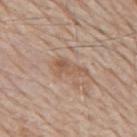A roughly 15 mm field-of-view crop from a total-body skin photograph. The lesion is on the mid back. A male patient, aged approximately 80. Approximately 4.5 mm at its widest. Automated image analysis of the tile measured a lesion area of about 6.5 mm², an eccentricity of roughly 0.9, and a symmetry-axis asymmetry near 0.45. The software also gave an average lesion color of about L≈56 a*≈18 b*≈30 (CIELAB), a lesion–skin lightness drop of about 8, and a normalized border contrast of about 6.